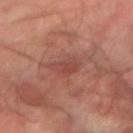Q: Is there a histopathology result?
A: total-body-photography surveillance lesion; no biopsy
Q: What kind of image is this?
A: 15 mm crop, total-body photography
Q: Who is the patient?
A: male, in their mid- to late 60s
Q: Where on the body is the lesion?
A: the right forearm